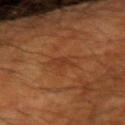Impression:
The lesion was photographed on a routine skin check and not biopsied; there is no pathology result.
Image and clinical context:
A male subject aged around 60. A 15 mm close-up tile from a total-body photography series done for melanoma screening. Measured at roughly 3 mm in maximum diameter. Captured under cross-polarized illumination. Located on the right forearm.